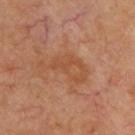notes: total-body-photography surveillance lesion; no biopsy, patient: aged around 65, acquisition: ~15 mm tile from a whole-body skin photo, anatomic site: the upper back.